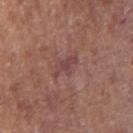<case>
<biopsy_status>not biopsied; imaged during a skin examination</biopsy_status>
<patient>
  <sex>male</sex>
  <age_approx>65</age_approx>
</patient>
<lighting>white-light</lighting>
<image>
  <source>total-body photography crop</source>
  <field_of_view_mm>15</field_of_view_mm>
</image>
<automated_metrics>
  <cielab_L>43</cielab_L>
  <cielab_a>22</cielab_a>
  <cielab_b>20</cielab_b>
  <vs_skin_darker_L>7.0</vs_skin_darker_L>
  <border_irregularity_0_10>5.5</border_irregularity_0_10>
  <color_variation_0_10>1.0</color_variation_0_10>
  <peripheral_color_asymmetry>0.5</peripheral_color_asymmetry>
  <nevus_likeness_0_100>0</nevus_likeness_0_100>
  <lesion_detection_confidence_0_100>90</lesion_detection_confidence_0_100>
</automated_metrics>
<lesion_size>
  <long_diameter_mm_approx>3.5</long_diameter_mm_approx>
</lesion_size>
<site>right upper arm</site>
</case>